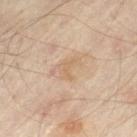Recorded during total-body skin imaging; not selected for excision or biopsy. The recorded lesion diameter is about 3 mm. The patient is approximately 55 years of age. A 15 mm crop from a total-body photograph taken for skin-cancer surveillance. From the leg. An algorithmic analysis of the crop reported a shape eccentricity near 0.8 and a symmetry-axis asymmetry near 0.55. It also reported a lesion color around L≈63 a*≈15 b*≈33 in CIELAB, roughly 6 lightness units darker than nearby skin, and a lesion-to-skin contrast of about 4.5 (normalized; higher = more distinct). The software also gave border irregularity of about 5.5 on a 0–10 scale and peripheral color asymmetry of about 0.5. The software also gave a nevus-likeness score of about 0/100. This is a cross-polarized tile.